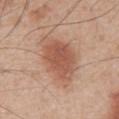On the abdomen. The patient is a male aged 53 to 57. Cropped from a whole-body photographic skin survey; the tile spans about 15 mm. An algorithmic analysis of the crop reported an area of roughly 22 mm², a shape eccentricity near 0.75, and a symmetry-axis asymmetry near 0.25. The analysis additionally found a lesion–skin lightness drop of about 11 and a normalized lesion–skin contrast near 7.5. It also reported border irregularity of about 2.5 on a 0–10 scale, a color-variation rating of about 3.5/10, and a peripheral color-asymmetry measure near 1. The software also gave an automated nevus-likeness rating near 100 out of 100. Approximately 7 mm at its widest.